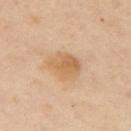anatomic site: the left arm
lighting: cross-polarized
image-analysis metrics: an area of roughly 8 mm², an outline eccentricity of about 0.45 (0 = round, 1 = elongated), and a symmetry-axis asymmetry near 0.2
subject: female, aged 38–42
image: total-body-photography crop, ~15 mm field of view